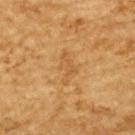Q: Was a biopsy performed?
A: catalogued during a skin exam; not biopsied
Q: How was this image acquired?
A: ~15 mm crop, total-body skin-cancer survey
Q: Illumination type?
A: cross-polarized
Q: What did automated image analysis measure?
A: a classifier nevus-likeness of about 0/100
Q: What is the anatomic site?
A: the upper back
Q: How large is the lesion?
A: ~4 mm (longest diameter)
Q: Patient demographics?
A: male, approximately 85 years of age or older